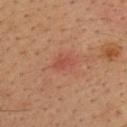Captured during whole-body skin photography for melanoma surveillance; the lesion was not biopsied. The lesion is located on the upper back. Automated tile analysis of the lesion measured an area of roughly 3 mm², an eccentricity of roughly 0.8, and a shape-asymmetry score of about 0.3 (0 = symmetric). And it measured border irregularity of about 3 on a 0–10 scale, a within-lesion color-variation index near 1/10, and peripheral color asymmetry of about 0.5. The software also gave a classifier nevus-likeness of about 0/100 and a detector confidence of about 100 out of 100 that the crop contains a lesion. A male patient, about 35 years old. Approximately 2.5 mm at its widest. A region of skin cropped from a whole-body photographic capture, roughly 15 mm wide.